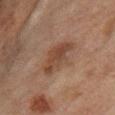Case summary:
– follow-up: imaged on a skin check; not biopsied
– body site: the leg
– image: ~15 mm crop, total-body skin-cancer survey
– illumination: cross-polarized illumination
– patient: female, aged around 55
– lesion size: about 5 mm